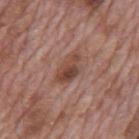No biopsy was performed on this lesion — it was imaged during a full skin examination and was not determined to be concerning. This image is a 15 mm lesion crop taken from a total-body photograph. Located on the mid back. Automated image analysis of the tile measured a footprint of about 6.5 mm², an outline eccentricity of about 0.85 (0 = round, 1 = elongated), and a shape-asymmetry score of about 0.25 (0 = symmetric). The analysis additionally found a lesion–skin lightness drop of about 10 and a normalized border contrast of about 8. The software also gave border irregularity of about 3 on a 0–10 scale and radial color variation of about 1.5. And it measured a lesion-detection confidence of about 100/100. Approximately 4 mm at its widest. The patient is a male roughly 70 years of age. Captured under white-light illumination.